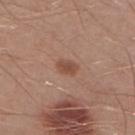follow-up = total-body-photography surveillance lesion; no biopsy
acquisition = ~15 mm crop, total-body skin-cancer survey
patient = male, aged approximately 30
body site = the right lower leg